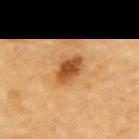Captured during whole-body skin photography for melanoma surveillance; the lesion was not biopsied. Imaged with cross-polarized lighting. From the left upper arm. A close-up tile cropped from a whole-body skin photograph, about 15 mm across. Approximately 4 mm at its widest. The patient is a male aged 83 or over. Automated image analysis of the tile measured a lesion area of about 7 mm² and a shape eccentricity near 0.8. And it measured border irregularity of about 2 on a 0–10 scale, a color-variation rating of about 3.5/10, and radial color variation of about 1. It also reported a classifier nevus-likeness of about 95/100 and a lesion-detection confidence of about 100/100.